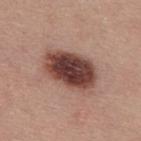site = the upper back
image-analysis metrics = a lesion area of about 20 mm², an eccentricity of roughly 0.8, and a symmetry-axis asymmetry near 0.15; a lesion color around L≈42 a*≈21 b*≈23 in CIELAB; an automated nevus-likeness rating near 75 out of 100 and a detector confidence of about 100 out of 100 that the crop contains a lesion
tile lighting = white-light
subject = male, aged approximately 40
image source = 15 mm crop, total-body photography